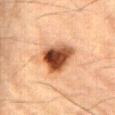Assessment: No biopsy was performed on this lesion — it was imaged during a full skin examination and was not determined to be concerning. Clinical summary: Automated image analysis of the tile measured a footprint of about 13 mm² and a shape-asymmetry score of about 0.3 (0 = symmetric). And it measured border irregularity of about 2.5 on a 0–10 scale, internal color variation of about 9 on a 0–10 scale, and a peripheral color-asymmetry measure near 2.5. It also reported a nevus-likeness score of about 95/100 and a lesion-detection confidence of about 100/100. From the abdomen. This is a cross-polarized tile. About 5 mm across. Cropped from a whole-body photographic skin survey; the tile spans about 15 mm. A male patient, roughly 85 years of age or older.